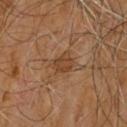Notes:
- notes: total-body-photography surveillance lesion; no biopsy